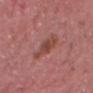biopsy status=catalogued during a skin exam; not biopsied
body site=the head or neck
imaging modality=~15 mm tile from a whole-body skin photo
subject=male, aged 63 to 67